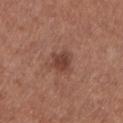{"biopsy_status": "not biopsied; imaged during a skin examination", "lighting": "white-light", "automated_metrics": {"area_mm2_approx": 5.0, "eccentricity": 0.45, "cielab_L": 41, "cielab_a": 23, "cielab_b": 26, "vs_skin_darker_L": 10.0, "vs_skin_contrast_norm": 8.0, "border_irregularity_0_10": 3.0, "color_variation_0_10": 2.0, "peripheral_color_asymmetry": 0.5}, "site": "leg", "image": {"source": "total-body photography crop", "field_of_view_mm": 15}, "patient": {"sex": "female", "age_approx": 55}, "lesion_size": {"long_diameter_mm_approx": 2.5}}